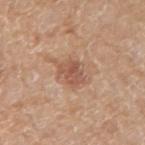No biopsy was performed on this lesion — it was imaged during a full skin examination and was not determined to be concerning. Imaged with white-light lighting. The recorded lesion diameter is about 3 mm. On the left forearm. A female subject, aged 73 to 77. Automated image analysis of the tile measured about 9 CIELAB-L* units darker than the surrounding skin and a normalized lesion–skin contrast near 6.5. The analysis additionally found a nevus-likeness score of about 20/100. A lesion tile, about 15 mm wide, cut from a 3D total-body photograph.